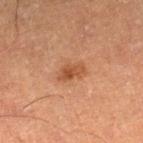Background:
About 3 mm across. This is a cross-polarized tile. The lesion-visualizer software estimated internal color variation of about 3.5 on a 0–10 scale and peripheral color asymmetry of about 1. A female subject in their 60s. A close-up tile cropped from a whole-body skin photograph, about 15 mm across. From the right lower leg.